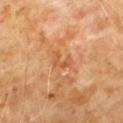Q: Was a biopsy performed?
A: total-body-photography surveillance lesion; no biopsy
Q: Lesion size?
A: ≈2.5 mm
Q: Who is the patient?
A: male, about 60 years old
Q: Lesion location?
A: the chest
Q: How was the tile lit?
A: cross-polarized illumination
Q: What kind of image is this?
A: total-body-photography crop, ~15 mm field of view